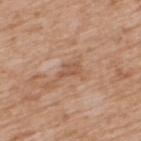Q: Is there a histopathology result?
A: catalogued during a skin exam; not biopsied
Q: What are the patient's age and sex?
A: male, aged around 65
Q: What is the anatomic site?
A: the upper back
Q: What lighting was used for the tile?
A: white-light illumination
Q: How large is the lesion?
A: ~3 mm (longest diameter)
Q: What kind of image is this?
A: ~15 mm crop, total-body skin-cancer survey
Q: What did automated image analysis measure?
A: an area of roughly 3.5 mm², a shape eccentricity near 0.85, and a symmetry-axis asymmetry near 0.4